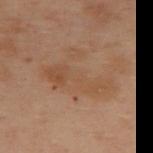Captured during whole-body skin photography for melanoma surveillance; the lesion was not biopsied.
A 15 mm close-up extracted from a 3D total-body photography capture.
The subject is a female approximately 55 years of age.
From the upper back.
Captured under cross-polarized illumination.